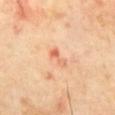<case>
<biopsy_status>not biopsied; imaged during a skin examination</biopsy_status>
<lighting>cross-polarized</lighting>
<image>
  <source>total-body photography crop</source>
  <field_of_view_mm>15</field_of_view_mm>
</image>
<patient>
  <sex>male</sex>
  <age_approx>65</age_approx>
</patient>
<automated_metrics>
  <area_mm2_approx>2.5</area_mm2_approx>
  <eccentricity>0.9</eccentricity>
  <cielab_L>68</cielab_L>
  <cielab_a>29</cielab_a>
  <cielab_b>37</cielab_b>
  <vs_skin_darker_L>10.0</vs_skin_darker_L>
  <vs_skin_contrast_norm>6.0</vs_skin_contrast_norm>
  <border_irregularity_0_10>6.5</border_irregularity_0_10>
  <color_variation_0_10>0.0</color_variation_0_10>
  <peripheral_color_asymmetry>0.0</peripheral_color_asymmetry>
</automated_metrics>
<site>back</site>
</case>